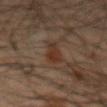  biopsy_status: not biopsied; imaged during a skin examination
  image:
    source: total-body photography crop
    field_of_view_mm: 15
  lesion_size:
    long_diameter_mm_approx: 3.0
  lighting: cross-polarized
  patient:
    sex: male
    age_approx: 50
  automated_metrics:
    cielab_L: 23
    cielab_a: 14
    cielab_b: 21
    vs_skin_darker_L: 6.0
    vs_skin_contrast_norm: 7.5
    border_irregularity_0_10: 3.0
    peripheral_color_asymmetry: 0.5
    nevus_likeness_0_100: 85
    lesion_detection_confidence_0_100: 100
  site: right forearm